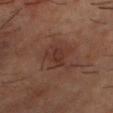| feature | finding |
|---|---|
| workup | no biopsy performed (imaged during a skin exam) |